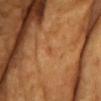| field | value |
|---|---|
| workup | catalogued during a skin exam; not biopsied |
| patient | female, aged 68 to 72 |
| site | the front of the torso |
| image | 15 mm crop, total-body photography |
| diameter | ~1.5 mm (longest diameter) |
| illumination | cross-polarized |
| automated metrics | a mean CIELAB color near L≈52 a*≈26 b*≈42, a lesion–skin lightness drop of about 5, and a normalized border contrast of about 4 |